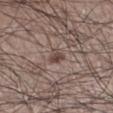lesion diameter — about 2.5 mm; TBP lesion metrics — an area of roughly 3 mm², an outline eccentricity of about 0.7 (0 = round, 1 = elongated), and a shape-asymmetry score of about 0.3 (0 = symmetric); lighting — white-light; patient — male, aged around 65; location — the left lower leg; image source — total-body-photography crop, ~15 mm field of view.